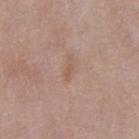Part of a total-body skin-imaging series; this lesion was reviewed on a skin check and was not flagged for biopsy.
A lesion tile, about 15 mm wide, cut from a 3D total-body photograph.
A male patient in their mid- to late 50s.
The recorded lesion diameter is about 2.5 mm.
The lesion is located on the front of the torso.